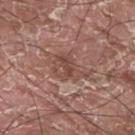Part of a total-body skin-imaging series; this lesion was reviewed on a skin check and was not flagged for biopsy. The lesion is on the upper back. A male patient, aged approximately 40. Measured at roughly 4 mm in maximum diameter. Captured under white-light illumination. A roughly 15 mm field-of-view crop from a total-body skin photograph.